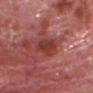Findings:
• notes · total-body-photography surveillance lesion; no biopsy
• image · 15 mm crop, total-body photography
• site · the head or neck
• image-analysis metrics · a footprint of about 7.5 mm², an outline eccentricity of about 0.8 (0 = round, 1 = elongated), and two-axis asymmetry of about 0.3; an average lesion color of about L≈40 a*≈30 b*≈27 (CIELAB) and a lesion–skin lightness drop of about 9; an automated nevus-likeness rating near 0 out of 100 and a lesion-detection confidence of about 100/100
• size · ≈4 mm
• tile lighting · white-light illumination
• patient · male, aged around 80